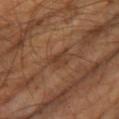Part of a total-body skin-imaging series; this lesion was reviewed on a skin check and was not flagged for biopsy. Cropped from a whole-body photographic skin survey; the tile spans about 15 mm. This is a cross-polarized tile. A male patient, aged approximately 65. Automated tile analysis of the lesion measured an average lesion color of about L≈37 a*≈19 b*≈29 (CIELAB), about 6 CIELAB-L* units darker than the surrounding skin, and a normalized lesion–skin contrast near 6.